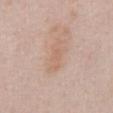Clinical impression: This lesion was catalogued during total-body skin photography and was not selected for biopsy. Context: The lesion is on the abdomen. A male subject, roughly 40 years of age. This is a white-light tile. A lesion tile, about 15 mm wide, cut from a 3D total-body photograph.